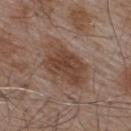A region of skin cropped from a whole-body photographic capture, roughly 15 mm wide. Captured under white-light illumination. From the upper back. Longest diameter approximately 6.5 mm. A male patient, aged 53 to 57.